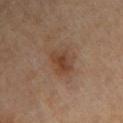| key | value |
|---|---|
| follow-up | catalogued during a skin exam; not biopsied |
| acquisition | ~15 mm crop, total-body skin-cancer survey |
| lesion size | ≈3.5 mm |
| TBP lesion metrics | a mean CIELAB color near L≈40 a*≈18 b*≈28, about 8 CIELAB-L* units darker than the surrounding skin, and a normalized border contrast of about 7; a border-irregularity rating of about 2.5/10 and a color-variation rating of about 4.5/10; a classifier nevus-likeness of about 20/100 and lesion-presence confidence of about 100/100 |
| tile lighting | cross-polarized illumination |
| location | the right upper arm |
| subject | male, aged 83 or over |